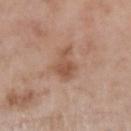notes — imaged on a skin check; not biopsied
location — the left upper arm
image — 15 mm crop, total-body photography
patient — male, aged 53 to 57